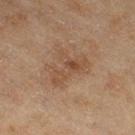Recorded during total-body skin imaging; not selected for excision or biopsy.
Located on the right thigh.
A female patient, aged 58–62.
The tile uses cross-polarized illumination.
Cropped from a total-body skin-imaging series; the visible field is about 15 mm.